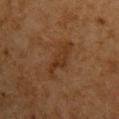No biopsy was performed on this lesion — it was imaged during a full skin examination and was not determined to be concerning. A 15 mm close-up tile from a total-body photography series done for melanoma screening. From the front of the torso. A male subject, aged around 60.